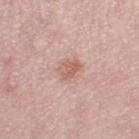Clinical summary: The lesion is on the leg. A 15 mm crop from a total-body photograph taken for skin-cancer surveillance. Automated image analysis of the tile measured a classifier nevus-likeness of about 30/100 and a lesion-detection confidence of about 100/100. The patient is a female approximately 50 years of age. Longest diameter approximately 3 mm.